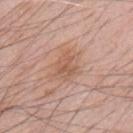On the mid back.
The tile uses white-light illumination.
The patient is a male roughly 60 years of age.
Cropped from a whole-body photographic skin survey; the tile spans about 15 mm.